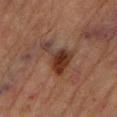This lesion was catalogued during total-body skin photography and was not selected for biopsy. The lesion's longest dimension is about 4.5 mm. The subject is a male in their mid- to late 80s or older. A region of skin cropped from a whole-body photographic capture, roughly 15 mm wide. The lesion is on the left lower leg.